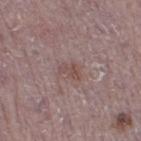Recorded during total-body skin imaging; not selected for excision or biopsy. A male subject aged 73 to 77. A region of skin cropped from a whole-body photographic capture, roughly 15 mm wide. Longest diameter approximately 2.5 mm. On the right thigh. An algorithmic analysis of the crop reported a lesion area of about 3 mm², an eccentricity of roughly 0.8, and two-axis asymmetry of about 0.45. The analysis additionally found a mean CIELAB color near L≈48 a*≈18 b*≈20 and about 7 CIELAB-L* units darker than the surrounding skin. Captured under white-light illumination.